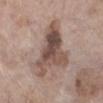Background: A female subject in their mid-70s. The recorded lesion diameter is about 7.5 mm. A region of skin cropped from a whole-body photographic capture, roughly 15 mm wide. Located on the leg.